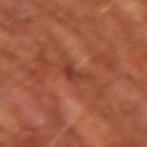Case summary:
- notes · imaged on a skin check; not biopsied
- subject · male, in their mid-60s
- size · ≈2.5 mm
- image source · 15 mm crop, total-body photography
- body site · the right upper arm